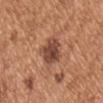Case summary:
– workup — catalogued during a skin exam; not biopsied
– lighting — white-light illumination
– site — the chest
– diameter — about 4 mm
– subject — male, in their mid-50s
– image source — ~15 mm crop, total-body skin-cancer survey